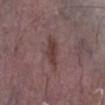Part of a total-body skin-imaging series; this lesion was reviewed on a skin check and was not flagged for biopsy.
The lesion is on the leg.
Imaged with white-light lighting.
Cropped from a whole-body photographic skin survey; the tile spans about 15 mm.
The patient is a male aged 38–42.
An algorithmic analysis of the crop reported a lesion color around L≈39 a*≈19 b*≈19 in CIELAB, roughly 8 lightness units darker than nearby skin, and a lesion-to-skin contrast of about 7.5 (normalized; higher = more distinct).
Approximately 4.5 mm at its widest.